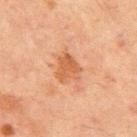Clinical impression:
The lesion was tiled from a total-body skin photograph and was not biopsied.
Clinical summary:
Captured under cross-polarized illumination. The lesion is on the front of the torso. A 15 mm crop from a total-body photograph taken for skin-cancer surveillance. A male patient aged 63–67.